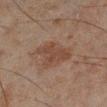workup: catalogued during a skin exam; not biopsied | acquisition: total-body-photography crop, ~15 mm field of view | patient: male, about 45 years old | automated lesion analysis: border irregularity of about 3.5 on a 0–10 scale, a within-lesion color-variation index near 2/10, and peripheral color asymmetry of about 0.5 | site: the right lower leg | tile lighting: cross-polarized | diameter: ~5 mm (longest diameter).